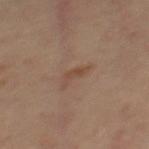Context:
Located on the back. About 3 mm across. A female subject aged approximately 45. The lesion-visualizer software estimated a footprint of about 3 mm² and a symmetry-axis asymmetry near 0.55. It also reported a lesion color around L≈44 a*≈17 b*≈27 in CIELAB, about 6 CIELAB-L* units darker than the surrounding skin, and a lesion-to-skin contrast of about 5.5 (normalized; higher = more distinct). And it measured a border-irregularity index near 6.5/10, internal color variation of about 0 on a 0–10 scale, and peripheral color asymmetry of about 0. The software also gave a classifier nevus-likeness of about 0/100. This is a cross-polarized tile. A close-up tile cropped from a whole-body skin photograph, about 15 mm across.